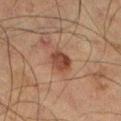biopsy status — catalogued during a skin exam; not biopsied | location — the left thigh | patient — male, aged 63–67 | lighting — cross-polarized illumination | image source — 15 mm crop, total-body photography | lesion size — ~2.5 mm (longest diameter) | TBP lesion metrics — a lesion area of about 5.5 mm² and a shape-asymmetry score of about 0.15 (0 = symmetric); a mean CIELAB color near L≈34 a*≈19 b*≈24 and a lesion–skin lightness drop of about 10; a border-irregularity index near 1.5/10 and radial color variation of about 1.5.